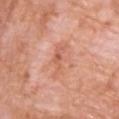This lesion was catalogued during total-body skin photography and was not selected for biopsy. The patient is a male approximately 60 years of age. The lesion is located on the chest. A lesion tile, about 15 mm wide, cut from a 3D total-body photograph.